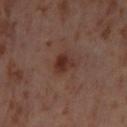| field | value |
|---|---|
| biopsy status | catalogued during a skin exam; not biopsied |
| location | the leg |
| lighting | cross-polarized |
| size | ≈2.5 mm |
| imaging modality | ~15 mm crop, total-body skin-cancer survey |
| subject | female, roughly 55 years of age |